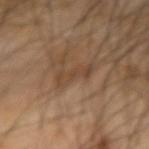Imaged during a routine full-body skin examination; the lesion was not biopsied and no histopathology is available.
On the right forearm.
This image is a 15 mm lesion crop taken from a total-body photograph.
A male patient in their mid- to late 60s.
Longest diameter approximately 4 mm.